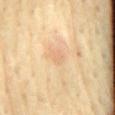follow-up = catalogued during a skin exam; not biopsied
anatomic site = the lower back
tile lighting = cross-polarized
automated metrics = a lesion color around L≈72 a*≈18 b*≈37 in CIELAB and a normalized lesion–skin contrast near 3.5
subject = male, about 70 years old
size = ≈2 mm
image source = ~15 mm crop, total-body skin-cancer survey